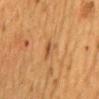Clinical impression:
Captured during whole-body skin photography for melanoma surveillance; the lesion was not biopsied.
Image and clinical context:
A female subject, aged approximately 50. The lesion is on the mid back. This image is a 15 mm lesion crop taken from a total-body photograph.